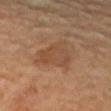biopsy status: catalogued during a skin exam; not biopsied | patient: female, roughly 70 years of age | site: the right upper arm | imaging modality: total-body-photography crop, ~15 mm field of view | size: ~4.5 mm (longest diameter) | automated metrics: an eccentricity of roughly 0.35 and a symmetry-axis asymmetry near 0.35; a lesion–skin lightness drop of about 6; a border-irregularity index near 3.5/10, a within-lesion color-variation index near 3.5/10, and a peripheral color-asymmetry measure near 1; a classifier nevus-likeness of about 10/100 | tile lighting: cross-polarized illumination.